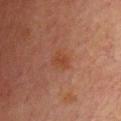patient — male, aged around 65; body site — the back; imaging modality — ~15 mm crop, total-body skin-cancer survey; diameter — about 2.5 mm.